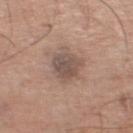- location · the left lower leg
- diameter · about 4 mm
- patient · male, approximately 60 years of age
- imaging modality · total-body-photography crop, ~15 mm field of view